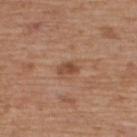The lesion was photographed on a routine skin check and not biopsied; there is no pathology result.
The patient is a male aged 63–67.
Cropped from a whole-body photographic skin survey; the tile spans about 15 mm.
On the upper back.